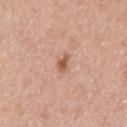Captured during whole-body skin photography for melanoma surveillance; the lesion was not biopsied. The subject is a male in their mid-50s. The total-body-photography lesion software estimated a lesion color around L≈57 a*≈23 b*≈31 in CIELAB, about 11 CIELAB-L* units darker than the surrounding skin, and a normalized lesion–skin contrast near 7.5. The software also gave border irregularity of about 3.5 on a 0–10 scale, a color-variation rating of about 2/10, and radial color variation of about 0.5. The lesion is located on the abdomen. Captured under white-light illumination. Longest diameter approximately 2.5 mm. A 15 mm crop from a total-body photograph taken for skin-cancer surveillance.